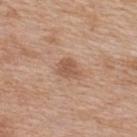biopsy_status: not biopsied; imaged during a skin examination
automated_metrics:
  cielab_L: 55
  cielab_a: 20
  cielab_b: 31
  vs_skin_darker_L: 9.0
  vs_skin_contrast_norm: 6.5
site: upper back
patient:
  sex: female
  age_approx: 40
image:
  source: total-body photography crop
  field_of_view_mm: 15